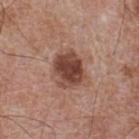Q: What is the imaging modality?
A: total-body-photography crop, ~15 mm field of view
Q: Illumination type?
A: white-light
Q: Lesion location?
A: the back
Q: What are the patient's age and sex?
A: male, about 65 years old